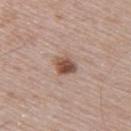Part of a total-body skin-imaging series; this lesion was reviewed on a skin check and was not flagged for biopsy.
A male patient roughly 50 years of age.
On the back.
Longest diameter approximately 2.5 mm.
Imaged with white-light lighting.
A 15 mm close-up extracted from a 3D total-body photography capture.